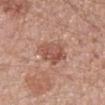| field | value |
|---|---|
| workup | imaged on a skin check; not biopsied |
| size | about 4 mm |
| patient | female, in their 50s |
| TBP lesion metrics | a border-irregularity rating of about 2.5/10 and a within-lesion color-variation index near 4/10; a classifier nevus-likeness of about 20/100 and a lesion-detection confidence of about 100/100 |
| imaging modality | total-body-photography crop, ~15 mm field of view |
| site | the left forearm |